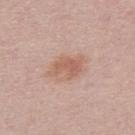No biopsy was performed on this lesion — it was imaged during a full skin examination and was not determined to be concerning.
A male patient, aged 28 to 32.
An algorithmic analysis of the crop reported an outline eccentricity of about 0.75 (0 = round, 1 = elongated) and a symmetry-axis asymmetry near 0.2.
Captured under white-light illumination.
Cropped from a total-body skin-imaging series; the visible field is about 15 mm.
The lesion's longest dimension is about 4.5 mm.